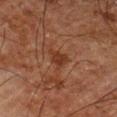* biopsy status · catalogued during a skin exam; not biopsied
* acquisition · ~15 mm crop, total-body skin-cancer survey
* subject · male, approximately 80 years of age
* automated lesion analysis · a lesion color around L≈27 a*≈19 b*≈26 in CIELAB, roughly 6 lightness units darker than nearby skin, and a normalized lesion–skin contrast near 7
* location · the left thigh
* lighting · cross-polarized illumination
* lesion diameter · ~2.5 mm (longest diameter)